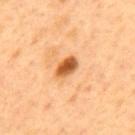follow-up: total-body-photography surveillance lesion; no biopsy | imaging modality: ~15 mm tile from a whole-body skin photo | patient: male, aged approximately 50 | site: the back.